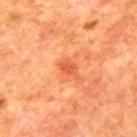biopsy_status: not biopsied; imaged during a skin examination
lighting: cross-polarized
site: back
image:
  source: total-body photography crop
  field_of_view_mm: 15
automated_metrics:
  area_mm2_approx: 3.5
  eccentricity: 0.75
  shape_asymmetry: 0.3
  border_irregularity_0_10: 3.0
  color_variation_0_10: 2.0
  peripheral_color_asymmetry: 0.5
  nevus_likeness_0_100: 0
patient:
  sex: male
  age_approx: 80
lesion_size:
  long_diameter_mm_approx: 3.0